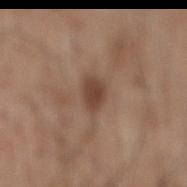Q: Is there a histopathology result?
A: imaged on a skin check; not biopsied
Q: How large is the lesion?
A: about 3 mm
Q: What did automated image analysis measure?
A: an area of roughly 5.5 mm², a shape eccentricity near 0.7, and two-axis asymmetry of about 0.25
Q: Patient demographics?
A: male, roughly 60 years of age
Q: What is the imaging modality?
A: total-body-photography crop, ~15 mm field of view
Q: Lesion location?
A: the back
Q: Illumination type?
A: white-light illumination